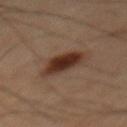Impression:
This lesion was catalogued during total-body skin photography and was not selected for biopsy.
Context:
The lesion is on the mid back. The subject is a male in their mid-40s. Imaged with cross-polarized lighting. Cropped from a total-body skin-imaging series; the visible field is about 15 mm.